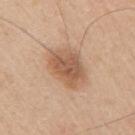Case summary:
– notes — total-body-photography surveillance lesion; no biopsy
– body site — the right upper arm
– patient — male, in their mid- to late 30s
– acquisition — 15 mm crop, total-body photography